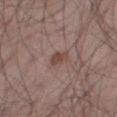Clinical impression: Part of a total-body skin-imaging series; this lesion was reviewed on a skin check and was not flagged for biopsy. Clinical summary: From the right thigh. An algorithmic analysis of the crop reported a symmetry-axis asymmetry near 0.3. And it measured a nevus-likeness score of about 45/100. Longest diameter approximately 2.5 mm. Cropped from a whole-body photographic skin survey; the tile spans about 15 mm. A male subject aged 53 to 57.